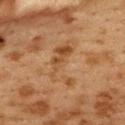biopsy status = no biopsy performed (imaged during a skin exam) | diameter = about 6 mm | tile lighting = cross-polarized illumination | acquisition = ~15 mm tile from a whole-body skin photo | anatomic site = the upper back | patient = female, approximately 40 years of age | automated metrics = a lesion area of about 13 mm² and two-axis asymmetry of about 0.55; a mean CIELAB color near L≈42 a*≈18 b*≈32 and about 5 CIELAB-L* units darker than the surrounding skin; a nevus-likeness score of about 0/100.